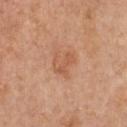Part of a total-body skin-imaging series; this lesion was reviewed on a skin check and was not flagged for biopsy. Approximately 3 mm at its widest. Located on the chest. A male patient aged 58 to 62. The tile uses white-light illumination. Cropped from a total-body skin-imaging series; the visible field is about 15 mm. Automated image analysis of the tile measured a lesion area of about 6 mm², a shape eccentricity near 0.65, and two-axis asymmetry of about 0.45. The analysis additionally found a mean CIELAB color near L≈57 a*≈23 b*≈35 and a normalized lesion–skin contrast near 5. It also reported a nevus-likeness score of about 20/100 and lesion-presence confidence of about 100/100.